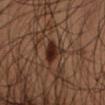  biopsy_status: not biopsied; imaged during a skin examination
  lesion_size:
    long_diameter_mm_approx: 3.0
  image:
    source: total-body photography crop
    field_of_view_mm: 15
  automated_metrics:
    cielab_L: 21
    cielab_a: 16
    cielab_b: 21
    vs_skin_darker_L: 10.0
    border_irregularity_0_10: 3.5
    color_variation_0_10: 3.0
    peripheral_color_asymmetry: 1.0
  lighting: cross-polarized
  patient:
    sex: male
    age_approx: 55
  site: arm